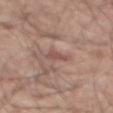A male patient, aged approximately 55. This image is a 15 mm lesion crop taken from a total-body photograph. Located on the mid back. The lesion's longest dimension is about 2.5 mm.